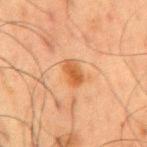follow-up: no biopsy performed (imaged during a skin exam)
patient: male, aged 58 to 62
diameter: ≈3.5 mm
illumination: cross-polarized illumination
automated metrics: a lesion area of about 5 mm² and two-axis asymmetry of about 0.2; a mean CIELAB color near L≈46 a*≈22 b*≈35, roughly 9 lightness units darker than nearby skin, and a normalized border contrast of about 8; a classifier nevus-likeness of about 80/100
site: the mid back
image source: ~15 mm tile from a whole-body skin photo